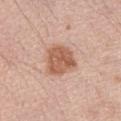Q: Is there a histopathology result?
A: no biopsy performed (imaged during a skin exam)
Q: How was this image acquired?
A: ~15 mm crop, total-body skin-cancer survey
Q: Lesion size?
A: ≈4 mm
Q: What is the anatomic site?
A: the front of the torso
Q: What are the patient's age and sex?
A: male, aged 68 to 72
Q: Illumination type?
A: white-light illumination
Q: Automated lesion metrics?
A: an average lesion color of about L≈58 a*≈23 b*≈31 (CIELAB), about 12 CIELAB-L* units darker than the surrounding skin, and a normalized border contrast of about 8.5; a border-irregularity index near 2.5/10, a color-variation rating of about 3.5/10, and radial color variation of about 1.5; a classifier nevus-likeness of about 65/100 and a detector confidence of about 100 out of 100 that the crop contains a lesion